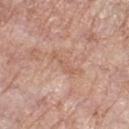No biopsy was performed on this lesion — it was imaged during a full skin examination and was not determined to be concerning.
A lesion tile, about 15 mm wide, cut from a 3D total-body photograph.
The lesion is on the right lower leg.
About 4 mm across.
The subject is a female approximately 70 years of age.
Automated image analysis of the tile measured a lesion color around L≈59 a*≈21 b*≈30 in CIELAB and about 7 CIELAB-L* units darker than the surrounding skin. It also reported border irregularity of about 6.5 on a 0–10 scale, a color-variation rating of about 0/10, and peripheral color asymmetry of about 0.